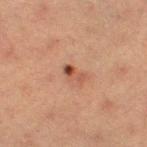Automated image analysis of the tile measured a lesion color around L≈42 a*≈21 b*≈27 in CIELAB, a lesion–skin lightness drop of about 10, and a lesion-to-skin contrast of about 8 (normalized; higher = more distinct). It also reported a nevus-likeness score of about 65/100 and a lesion-detection confidence of about 100/100.
The recorded lesion diameter is about 2.5 mm.
The patient is a female approximately 40 years of age.
A 15 mm crop from a total-body photograph taken for skin-cancer surveillance.
The lesion is on the leg.
The tile uses cross-polarized illumination.